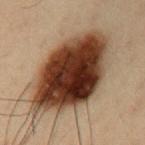Q: Was this lesion biopsied?
A: total-body-photography surveillance lesion; no biopsy
Q: How was the tile lit?
A: cross-polarized illumination
Q: Who is the patient?
A: male, aged 33 to 37
Q: How large is the lesion?
A: about 11 mm
Q: How was this image acquired?
A: ~15 mm tile from a whole-body skin photo
Q: What is the anatomic site?
A: the left upper arm